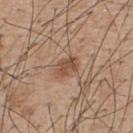notes: total-body-photography surveillance lesion; no biopsy
TBP lesion metrics: an eccentricity of roughly 0.8 and a shape-asymmetry score of about 0.2 (0 = symmetric); about 10 CIELAB-L* units darker than the surrounding skin and a lesion-to-skin contrast of about 7.5 (normalized; higher = more distinct); a classifier nevus-likeness of about 90/100 and a lesion-detection confidence of about 100/100
tile lighting: white-light illumination
patient: male, aged 53–57
site: the back
acquisition: total-body-photography crop, ~15 mm field of view